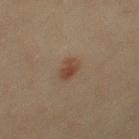Assessment:
The lesion was photographed on a routine skin check and not biopsied; there is no pathology result.
Acquisition and patient details:
The total-body-photography lesion software estimated a mean CIELAB color near L≈35 a*≈16 b*≈24, a lesion–skin lightness drop of about 8, and a lesion-to-skin contrast of about 7.5 (normalized; higher = more distinct). The software also gave a nevus-likeness score of about 95/100. This is a cross-polarized tile. Located on the right thigh. About 2 mm across. A 15 mm close-up extracted from a 3D total-body photography capture. A female patient aged 38 to 42.